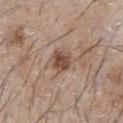{
  "biopsy_status": "not biopsied; imaged during a skin examination",
  "patient": {
    "sex": "male",
    "age_approx": 65
  },
  "lesion_size": {
    "long_diameter_mm_approx": 2.5
  },
  "image": {
    "source": "total-body photography crop",
    "field_of_view_mm": 15
  },
  "site": "front of the torso",
  "lighting": "white-light"
}